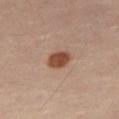Impression:
The lesion was tiled from a total-body skin photograph and was not biopsied.
Background:
This is a cross-polarized tile. The subject is a female aged around 60. The lesion's longest dimension is about 3 mm. Cropped from a total-body skin-imaging series; the visible field is about 15 mm. The lesion is on the right thigh. An algorithmic analysis of the crop reported an area of roughly 6 mm², an outline eccentricity of about 0.65 (0 = round, 1 = elongated), and two-axis asymmetry of about 0.2. The analysis additionally found an average lesion color of about L≈49 a*≈22 b*≈31 (CIELAB), roughly 14 lightness units darker than nearby skin, and a normalized lesion–skin contrast near 10.5. It also reported a within-lesion color-variation index near 2/10 and a peripheral color-asymmetry measure near 0.5.